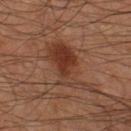Captured during whole-body skin photography for melanoma surveillance; the lesion was not biopsied. The recorded lesion diameter is about 7.5 mm. A region of skin cropped from a whole-body photographic capture, roughly 15 mm wide. A male patient, approximately 60 years of age. The tile uses cross-polarized illumination. The lesion is located on the right thigh.